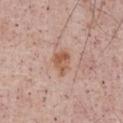Impression: This lesion was catalogued during total-body skin photography and was not selected for biopsy. Context: About 3 mm across. On the abdomen. The tile uses white-light illumination. Automated image analysis of the tile measured a mean CIELAB color near L≈57 a*≈22 b*≈30 and about 10 CIELAB-L* units darker than the surrounding skin. The software also gave a classifier nevus-likeness of about 25/100 and a lesion-detection confidence of about 100/100. The subject is a male roughly 55 years of age. Cropped from a whole-body photographic skin survey; the tile spans about 15 mm.